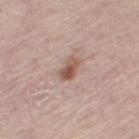Captured during whole-body skin photography for melanoma surveillance; the lesion was not biopsied. Located on the right thigh. The patient is a female in their mid-60s. The lesion-visualizer software estimated an eccentricity of roughly 0.85 and a symmetry-axis asymmetry near 0.25. It also reported roughly 12 lightness units darker than nearby skin and a normalized border contrast of about 8.5. It also reported border irregularity of about 2.5 on a 0–10 scale, a color-variation rating of about 6/10, and a peripheral color-asymmetry measure near 2. And it measured a classifier nevus-likeness of about 80/100 and a detector confidence of about 100 out of 100 that the crop contains a lesion. A region of skin cropped from a whole-body photographic capture, roughly 15 mm wide.